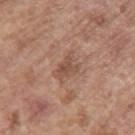biopsy status — imaged on a skin check; not biopsied
subject — female, aged 63–67
body site — the arm
lesion diameter — ~3 mm (longest diameter)
acquisition — ~15 mm tile from a whole-body skin photo